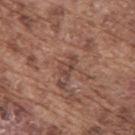Impression:
Imaged during a routine full-body skin examination; the lesion was not biopsied and no histopathology is available.
Clinical summary:
A male subject, about 75 years old. The lesion's longest dimension is about 3 mm. Located on the upper back. Automated tile analysis of the lesion measured border irregularity of about 5 on a 0–10 scale and internal color variation of about 2.5 on a 0–10 scale. The analysis additionally found an automated nevus-likeness rating near 0 out of 100 and lesion-presence confidence of about 60/100. A close-up tile cropped from a whole-body skin photograph, about 15 mm across.